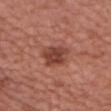Captured during whole-body skin photography for melanoma surveillance; the lesion was not biopsied.
Longest diameter approximately 3.5 mm.
This image is a 15 mm lesion crop taken from a total-body photograph.
On the front of the torso.
The total-body-photography lesion software estimated an area of roughly 9 mm², an outline eccentricity of about 0.65 (0 = round, 1 = elongated), and two-axis asymmetry of about 0.2. The software also gave a mean CIELAB color near L≈44 a*≈26 b*≈29.
A male patient roughly 75 years of age.